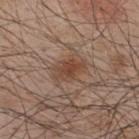Recorded during total-body skin imaging; not selected for excision or biopsy. The lesion-visualizer software estimated a lesion color around L≈44 a*≈19 b*≈28 in CIELAB, about 9 CIELAB-L* units darker than the surrounding skin, and a lesion-to-skin contrast of about 7.5 (normalized; higher = more distinct). The software also gave a classifier nevus-likeness of about 80/100 and a detector confidence of about 100 out of 100 that the crop contains a lesion. This is a white-light tile. About 3.5 mm across. A region of skin cropped from a whole-body photographic capture, roughly 15 mm wide. Located on the upper back. A male subject, aged 48 to 52.